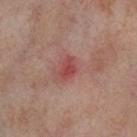lighting: cross-polarized
imaging modality: ~15 mm tile from a whole-body skin photo
location: the right thigh
automated lesion analysis: an average lesion color of about L≈47 a*≈30 b*≈24 (CIELAB) and a lesion-to-skin contrast of about 7 (normalized; higher = more distinct); border irregularity of about 3.5 on a 0–10 scale, a color-variation rating of about 2.5/10, and radial color variation of about 1; an automated nevus-likeness rating near 0 out of 100 and a detector confidence of about 100 out of 100 that the crop contains a lesion
patient: female, about 55 years old
diameter: ≈2.5 mm